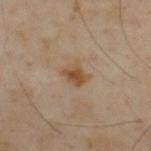No biopsy was performed on this lesion — it was imaged during a full skin examination and was not determined to be concerning.
The total-body-photography lesion software estimated border irregularity of about 3 on a 0–10 scale, a within-lesion color-variation index near 3.5/10, and a peripheral color-asymmetry measure near 1.
Approximately 3 mm at its widest.
Imaged with cross-polarized lighting.
A male patient roughly 55 years of age.
The lesion is on the upper back.
Cropped from a whole-body photographic skin survey; the tile spans about 15 mm.